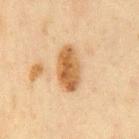biopsy_status: not biopsied; imaged during a skin examination
site: chest
image:
  source: total-body photography crop
  field_of_view_mm: 15
lesion_size:
  long_diameter_mm_approx: 5.0
lighting: cross-polarized
patient:
  sex: male
  age_approx: 60